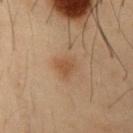The lesion was tiled from a total-body skin photograph and was not biopsied.
A close-up tile cropped from a whole-body skin photograph, about 15 mm across.
The subject is a male in their mid-50s.
The lesion is on the abdomen.
About 3 mm across.
Imaged with cross-polarized lighting.